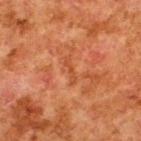size: ≈3 mm
image source: ~15 mm tile from a whole-body skin photo
patient: male, aged around 80
tile lighting: cross-polarized illumination
body site: the upper back